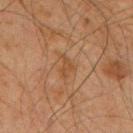Assessment:
Imaged during a routine full-body skin examination; the lesion was not biopsied and no histopathology is available.
Background:
A close-up tile cropped from a whole-body skin photograph, about 15 mm across. Located on the mid back. The patient is a male about 65 years old. The lesion's longest dimension is about 2.5 mm. This is a cross-polarized tile.